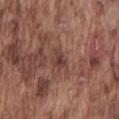Impression: Captured during whole-body skin photography for melanoma surveillance; the lesion was not biopsied. Context: This is a white-light tile. A male subject approximately 75 years of age. The recorded lesion diameter is about 2.5 mm. The total-body-photography lesion software estimated border irregularity of about 3.5 on a 0–10 scale, a within-lesion color-variation index near 3.5/10, and radial color variation of about 1.5. Cropped from a total-body skin-imaging series; the visible field is about 15 mm. From the mid back.